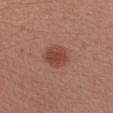The lesion was tiled from a total-body skin photograph and was not biopsied. The recorded lesion diameter is about 3.5 mm. The subject is a male aged approximately 30. This image is a 15 mm lesion crop taken from a total-body photograph. On the left upper arm. The tile uses white-light illumination.